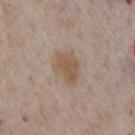Approximately 4 mm at its widest.
The total-body-photography lesion software estimated an automated nevus-likeness rating near 60 out of 100 and lesion-presence confidence of about 100/100.
Imaged with white-light lighting.
The lesion is located on the chest.
A 15 mm crop from a total-body photograph taken for skin-cancer surveillance.
A male patient roughly 75 years of age.